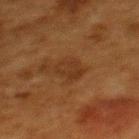workup — catalogued during a skin exam; not biopsied | body site — the upper back | automated metrics — a lesion color around L≈28 a*≈18 b*≈28 in CIELAB, roughly 5 lightness units darker than nearby skin, and a lesion-to-skin contrast of about 6 (normalized; higher = more distinct); a border-irregularity index near 3/10, a within-lesion color-variation index near 2.5/10, and radial color variation of about 1; an automated nevus-likeness rating near 0 out of 100 | lesion diameter — about 3 mm | subject — female, in their 50s | imaging modality — 15 mm crop, total-body photography.